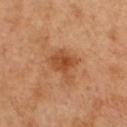workup: imaged on a skin check; not biopsied
diameter: ~4 mm (longest diameter)
patient: male, in their 50s
lighting: cross-polarized illumination
TBP lesion metrics: an area of roughly 9 mm², an outline eccentricity of about 0.65 (0 = round, 1 = elongated), and two-axis asymmetry of about 0.4; a border-irregularity rating of about 4.5/10 and a color-variation rating of about 3.5/10
anatomic site: the chest
image: ~15 mm tile from a whole-body skin photo